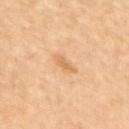{
  "biopsy_status": "not biopsied; imaged during a skin examination",
  "image": {
    "source": "total-body photography crop",
    "field_of_view_mm": 15
  },
  "site": "upper back",
  "lighting": "cross-polarized",
  "lesion_size": {
    "long_diameter_mm_approx": 2.5
  },
  "automated_metrics": {
    "area_mm2_approx": 2.0,
    "shape_asymmetry": 0.45,
    "cielab_L": 65,
    "cielab_a": 21,
    "cielab_b": 41,
    "vs_skin_darker_L": 8.0,
    "vs_skin_contrast_norm": 6.0,
    "lesion_detection_confidence_0_100": 100
  },
  "patient": {
    "sex": "female",
    "age_approx": 50
  }
}